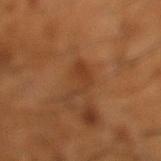Q: Was a biopsy performed?
A: total-body-photography surveillance lesion; no biopsy
Q: How was the tile lit?
A: cross-polarized
Q: Lesion location?
A: the left lower leg
Q: What are the patient's age and sex?
A: male, roughly 65 years of age
Q: What is the imaging modality?
A: total-body-photography crop, ~15 mm field of view
Q: Automated lesion metrics?
A: a footprint of about 5.5 mm² and an outline eccentricity of about 0.85 (0 = round, 1 = elongated); a mean CIELAB color near L≈38 a*≈22 b*≈34, a lesion–skin lightness drop of about 6, and a normalized border contrast of about 5; a border-irregularity rating of about 9/10 and peripheral color asymmetry of about 0.5; a lesion-detection confidence of about 100/100
Q: How large is the lesion?
A: ~4.5 mm (longest diameter)